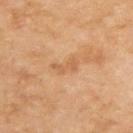workup: no biopsy performed (imaged during a skin exam)
tile lighting: cross-polarized
subject: male, in their mid-50s
anatomic site: the upper back
imaging modality: ~15 mm crop, total-body skin-cancer survey
diameter: about 3.5 mm